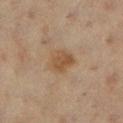Located on the left lower leg.
Automated tile analysis of the lesion measured a footprint of about 8 mm². The analysis additionally found a mean CIELAB color near L≈44 a*≈15 b*≈29, about 7 CIELAB-L* units darker than the surrounding skin, and a normalized lesion–skin contrast near 7. The software also gave border irregularity of about 2 on a 0–10 scale, a within-lesion color-variation index near 3/10, and radial color variation of about 1. And it measured an automated nevus-likeness rating near 45 out of 100 and lesion-presence confidence of about 100/100.
The lesion's longest dimension is about 3.5 mm.
A 15 mm close-up extracted from a 3D total-body photography capture.
A female subject aged around 55.
This is a cross-polarized tile.